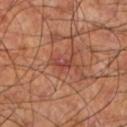Clinical impression:
Part of a total-body skin-imaging series; this lesion was reviewed on a skin check and was not flagged for biopsy.
Context:
Located on the leg. The subject is a male aged 58 to 62. The lesion's longest dimension is about 2.5 mm. Imaged with cross-polarized lighting. Cropped from a whole-body photographic skin survey; the tile spans about 15 mm.